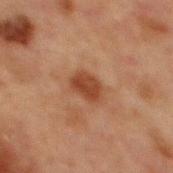{"biopsy_status": "not biopsied; imaged during a skin examination", "lighting": "cross-polarized", "image": {"source": "total-body photography crop", "field_of_view_mm": 15}, "automated_metrics": {"area_mm2_approx": 6.0, "eccentricity": 0.75, "shape_asymmetry": 0.2, "border_irregularity_0_10": 2.0, "peripheral_color_asymmetry": 1.0}, "lesion_size": {"long_diameter_mm_approx": 3.5}, "site": "chest", "patient": {"sex": "male", "age_approx": 65}}